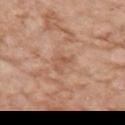The lesion was tiled from a total-body skin photograph and was not biopsied.
A lesion tile, about 15 mm wide, cut from a 3D total-body photograph.
Measured at roughly 2.5 mm in maximum diameter.
This is a white-light tile.
The patient is a female about 75 years old.
Automated tile analysis of the lesion measured an average lesion color of about L≈55 a*≈22 b*≈31 (CIELAB) and roughly 7 lightness units darker than nearby skin. And it measured a classifier nevus-likeness of about 0/100.
On the right upper arm.